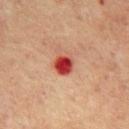Notes:
• notes — catalogued during a skin exam; not biopsied
• patient — male, in their mid-60s
• location — the abdomen
• tile lighting — cross-polarized
• image source — 15 mm crop, total-body photography
• size — ~3 mm (longest diameter)